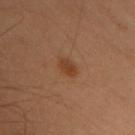Measured at roughly 2.5 mm in maximum diameter. Imaged with cross-polarized lighting. A female patient, aged 48 to 52. On the chest. Automated tile analysis of the lesion measured an average lesion color of about L≈34 a*≈18 b*≈29 (CIELAB) and a normalized lesion–skin contrast near 7. It also reported a within-lesion color-variation index near 2/10 and a peripheral color-asymmetry measure near 1. This image is a 15 mm lesion crop taken from a total-body photograph.